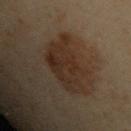{
  "biopsy_status": "not biopsied; imaged during a skin examination",
  "image": {
    "source": "total-body photography crop",
    "field_of_view_mm": 15
  },
  "site": "left upper arm",
  "lighting": "cross-polarized",
  "patient": {
    "sex": "male",
    "age_approx": 55
  },
  "lesion_size": {
    "long_diameter_mm_approx": 6.0
  }
}